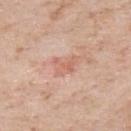Part of a total-body skin-imaging series; this lesion was reviewed on a skin check and was not flagged for biopsy. A male patient in their 70s. A 15 mm close-up tile from a total-body photography series done for melanoma screening. From the chest.